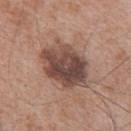Assessment:
The lesion was tiled from a total-body skin photograph and was not biopsied.
Image and clinical context:
Imaged with white-light lighting. This image is a 15 mm lesion crop taken from a total-body photograph. The lesion is on the upper back. The patient is a male roughly 65 years of age. Longest diameter approximately 6.5 mm.